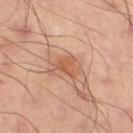illumination: cross-polarized illumination
anatomic site: the right thigh
TBP lesion metrics: a lesion area of about 4.5 mm² and a shape-asymmetry score of about 0.4 (0 = symmetric); a lesion color around L≈58 a*≈26 b*≈35 in CIELAB, a lesion–skin lightness drop of about 8, and a normalized lesion–skin contrast near 6.5; border irregularity of about 4 on a 0–10 scale and a color-variation rating of about 3/10
diameter: ≈2.5 mm
image source: ~15 mm crop, total-body skin-cancer survey